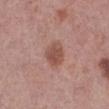Captured during whole-body skin photography for melanoma surveillance; the lesion was not biopsied. Cropped from a whole-body photographic skin survey; the tile spans about 15 mm. Captured under white-light illumination. The lesion is on the right lower leg. Longest diameter approximately 3 mm. A female patient approximately 40 years of age.